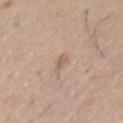notes: imaged on a skin check; not biopsied | anatomic site: the chest | automated lesion analysis: a border-irregularity index near 4/10 and peripheral color asymmetry of about 0 | subject: male, aged 28 to 32 | image source: ~15 mm tile from a whole-body skin photo | illumination: white-light illumination.